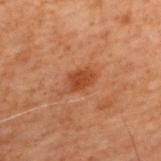Assessment:
Recorded during total-body skin imaging; not selected for excision or biopsy.
Context:
A roughly 15 mm field-of-view crop from a total-body skin photograph. This is a cross-polarized tile. On the upper back. A male patient aged 68–72.